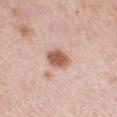Notes:
* workup: catalogued during a skin exam; not biopsied
* site: the left forearm
* imaging modality: ~15 mm tile from a whole-body skin photo
* subject: female, aged 48 to 52